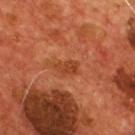biopsy status: catalogued during a skin exam; not biopsied | acquisition: ~15 mm crop, total-body skin-cancer survey | location: the chest | tile lighting: cross-polarized | subject: male, in their mid-50s.